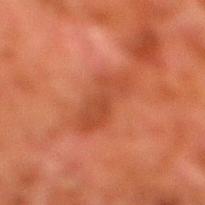Assessment: This lesion was catalogued during total-body skin photography and was not selected for biopsy. Image and clinical context: A male patient, aged 78–82. Cropped from a whole-body photographic skin survey; the tile spans about 15 mm. The lesion is located on the right lower leg.